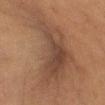The lesion was photographed on a routine skin check and not biopsied; there is no pathology result. The subject is a female approximately 50 years of age. The lesion is located on the left thigh. Approximately 13.5 mm at its widest. Automated image analysis of the tile measured a footprint of about 49 mm² and an eccentricity of roughly 0.85. And it measured border irregularity of about 8.5 on a 0–10 scale, a within-lesion color-variation index near 4/10, and peripheral color asymmetry of about 1. The analysis additionally found an automated nevus-likeness rating near 0 out of 100 and a lesion-detection confidence of about 60/100. A 15 mm close-up tile from a total-body photography series done for melanoma screening.